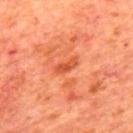The lesion was tiled from a total-body skin photograph and was not biopsied.
A male subject in their mid-60s.
Imaged with cross-polarized lighting.
A 15 mm crop from a total-body photograph taken for skin-cancer surveillance.
From the mid back.
The lesion's longest dimension is about 3 mm.